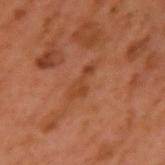workup = imaged on a skin check; not biopsied | patient = male, roughly 60 years of age | location = the left upper arm | imaging modality = ~15 mm tile from a whole-body skin photo.